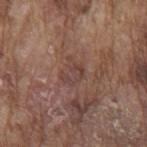{"biopsy_status": "not biopsied; imaged during a skin examination", "image": {"source": "total-body photography crop", "field_of_view_mm": 15}, "site": "mid back", "patient": {"sex": "male", "age_approx": 75}, "automated_metrics": {"area_mm2_approx": 4.0, "eccentricity": 0.6, "shape_asymmetry": 0.5, "cielab_L": 42, "cielab_a": 19, "cielab_b": 22, "vs_skin_darker_L": 7.0, "color_variation_0_10": 1.5, "peripheral_color_asymmetry": 0.5}, "lighting": "white-light", "lesion_size": {"long_diameter_mm_approx": 3.0}}